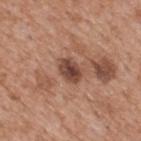biopsy status: catalogued during a skin exam; not biopsied | patient: male, roughly 65 years of age | body site: the mid back | tile lighting: white-light illumination | imaging modality: ~15 mm crop, total-body skin-cancer survey | automated metrics: an average lesion color of about L≈44 a*≈22 b*≈26 (CIELAB), roughly 13 lightness units darker than nearby skin, and a normalized border contrast of about 10 | size: ≈3 mm.